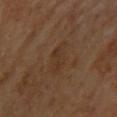Q: Was a biopsy performed?
A: imaged on a skin check; not biopsied
Q: What are the patient's age and sex?
A: female, in their 70s
Q: How was this image acquired?
A: total-body-photography crop, ~15 mm field of view
Q: Lesion location?
A: the upper back
Q: Automated lesion metrics?
A: a border-irregularity index near 3/10, internal color variation of about 2.5 on a 0–10 scale, and a peripheral color-asymmetry measure near 1
Q: Lesion size?
A: ~4.5 mm (longest diameter)
Q: What lighting was used for the tile?
A: cross-polarized